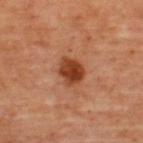{"lesion_size": {"long_diameter_mm_approx": 3.5}, "patient": {"sex": "female", "age_approx": 45}, "site": "upper back", "image": {"source": "total-body photography crop", "field_of_view_mm": 15}, "automated_metrics": {"cielab_L": 41, "cielab_a": 27, "cielab_b": 35, "vs_skin_darker_L": 13.0, "vs_skin_contrast_norm": 10.5, "color_variation_0_10": 4.5, "nevus_likeness_0_100": 95, "lesion_detection_confidence_0_100": 100}, "lighting": "cross-polarized"}